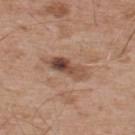Impression: This lesion was catalogued during total-body skin photography and was not selected for biopsy. Acquisition and patient details: The lesion's longest dimension is about 5 mm. A male patient, roughly 55 years of age. This image is a 15 mm lesion crop taken from a total-body photograph. Imaged with white-light lighting. On the back. The total-body-photography lesion software estimated a lesion area of about 8 mm², a shape eccentricity near 0.95, and a shape-asymmetry score of about 0.2 (0 = symmetric). It also reported a lesion color around L≈48 a*≈19 b*≈28 in CIELAB, about 13 CIELAB-L* units darker than the surrounding skin, and a lesion-to-skin contrast of about 9 (normalized; higher = more distinct). It also reported peripheral color asymmetry of about 5.